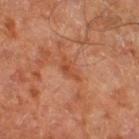Impression: This lesion was catalogued during total-body skin photography and was not selected for biopsy. Context: A patient aged approximately 65. Measured at roughly 3.5 mm in maximum diameter. An algorithmic analysis of the crop reported a mean CIELAB color near L≈49 a*≈27 b*≈36, a lesion–skin lightness drop of about 7, and a lesion-to-skin contrast of about 6 (normalized; higher = more distinct). The analysis additionally found a classifier nevus-likeness of about 0/100 and a detector confidence of about 100 out of 100 that the crop contains a lesion. Cropped from a whole-body photographic skin survey; the tile spans about 15 mm. Located on the right thigh.